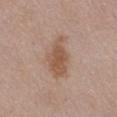Q: Is there a histopathology result?
A: imaged on a skin check; not biopsied
Q: What is the anatomic site?
A: the abdomen
Q: Who is the patient?
A: female, aged 68 to 72
Q: What is the lesion's diameter?
A: ~5 mm (longest diameter)
Q: What lighting was used for the tile?
A: white-light
Q: What did automated image analysis measure?
A: a lesion area of about 11 mm², an outline eccentricity of about 0.85 (0 = round, 1 = elongated), and two-axis asymmetry of about 0.3; an average lesion color of about L≈54 a*≈19 b*≈30 (CIELAB) and a normalized border contrast of about 7.5; a border-irregularity index near 3.5/10, a color-variation rating of about 2.5/10, and radial color variation of about 0.5
Q: What kind of image is this?
A: ~15 mm tile from a whole-body skin photo